The tile uses white-light illumination; The lesion-visualizer software estimated a lesion-detection confidence of about 95/100; approximately 11.5 mm at its widest; a male subject, in their 70s; on the left lower leg; a 15 mm close-up extracted from a 3D total-body photography capture — 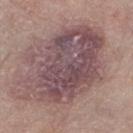Conclusion:
Histopathologically confirmed as a superficial basal cell carcinoma — a malignancy.